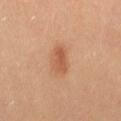No biopsy was performed on this lesion — it was imaged during a full skin examination and was not determined to be concerning.